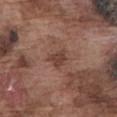{
  "biopsy_status": "not biopsied; imaged during a skin examination",
  "image": {
    "source": "total-body photography crop",
    "field_of_view_mm": 15
  },
  "lighting": "white-light",
  "lesion_size": {
    "long_diameter_mm_approx": 2.5
  },
  "site": "abdomen",
  "patient": {
    "sex": "male",
    "age_approx": 75
  }
}